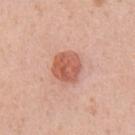Part of a total-body skin-imaging series; this lesion was reviewed on a skin check and was not flagged for biopsy. From the abdomen. Captured under white-light illumination. A close-up tile cropped from a whole-body skin photograph, about 15 mm across. The subject is a male approximately 55 years of age.